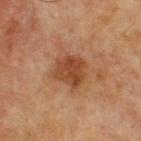  biopsy_status: not biopsied; imaged during a skin examination
  patient:
    sex: male
    age_approx: 65
  lesion_size:
    long_diameter_mm_approx: 4.0
  automated_metrics:
    area_mm2_approx: 11.0
    eccentricity: 0.5
    shape_asymmetry: 0.15
    cielab_L: 35
    cielab_a: 20
    cielab_b: 29
    vs_skin_darker_L: 8.0
    vs_skin_contrast_norm: 8.0
  site: chest
  image:
    source: total-body photography crop
    field_of_view_mm: 15
  lighting: cross-polarized